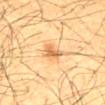| feature | finding |
|---|---|
| follow-up | catalogued during a skin exam; not biopsied |
| subject | male, roughly 60 years of age |
| lesion size | ~2.5 mm (longest diameter) |
| site | the abdomen |
| lighting | cross-polarized illumination |
| image | total-body-photography crop, ~15 mm field of view |